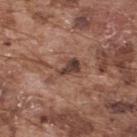{
  "biopsy_status": "not biopsied; imaged during a skin examination",
  "site": "back",
  "patient": {
    "sex": "male",
    "age_approx": 75
  },
  "image": {
    "source": "total-body photography crop",
    "field_of_view_mm": 15
  },
  "lighting": "white-light",
  "lesion_size": {
    "long_diameter_mm_approx": 5.0
  },
  "automated_metrics": {
    "border_irregularity_0_10": 6.5,
    "peripheral_color_asymmetry": 1.5
  }
}